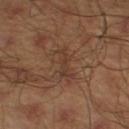biopsy_status: not biopsied; imaged during a skin examination
image:
  source: total-body photography crop
  field_of_view_mm: 15
automated_metrics:
  area_mm2_approx: 5.0
  shape_asymmetry: 0.35
  color_variation_0_10: 0.5
patient:
  sex: male
  age_approx: 45
lesion_size:
  long_diameter_mm_approx: 4.5
site: left lower leg
lighting: cross-polarized